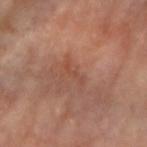biopsy status = no biopsy performed (imaged during a skin exam) | body site = the right forearm | diameter = ~2.5 mm (longest diameter) | patient = female, approximately 65 years of age | acquisition = ~15 mm crop, total-body skin-cancer survey.